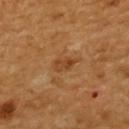Clinical impression: The lesion was photographed on a routine skin check and not biopsied; there is no pathology result. Background: About 3 mm across. The lesion is on the upper back. A region of skin cropped from a whole-body photographic capture, roughly 15 mm wide. A female subject, in their mid- to late 50s. Automated image analysis of the tile measured a mean CIELAB color near L≈45 a*≈23 b*≈39, a lesion–skin lightness drop of about 8, and a normalized lesion–skin contrast near 6.5. The analysis additionally found an automated nevus-likeness rating near 0 out of 100 and a detector confidence of about 100 out of 100 that the crop contains a lesion.